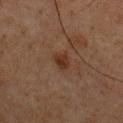The lesion was photographed on a routine skin check and not biopsied; there is no pathology result. The lesion's longest dimension is about 2.5 mm. Located on the front of the torso. Automated tile analysis of the lesion measured an area of roughly 4 mm² and a symmetry-axis asymmetry near 0.25. The analysis additionally found a lesion color around L≈26 a*≈16 b*≈24 in CIELAB, roughly 6 lightness units darker than nearby skin, and a lesion-to-skin contrast of about 7.5 (normalized; higher = more distinct). It also reported border irregularity of about 2.5 on a 0–10 scale and peripheral color asymmetry of about 0.5. The analysis additionally found a lesion-detection confidence of about 100/100. This image is a 15 mm lesion crop taken from a total-body photograph. A male patient, aged 73 to 77. This is a cross-polarized tile.